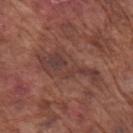<case>
  <lighting>white-light</lighting>
  <site>arm</site>
  <lesion_size>
    <long_diameter_mm_approx>8.0</long_diameter_mm_approx>
  </lesion_size>
  <image>
    <source>total-body photography crop</source>
    <field_of_view_mm>15</field_of_view_mm>
  </image>
  <patient>
    <sex>male</sex>
    <age_approx>75</age_approx>
  </patient>
</case>